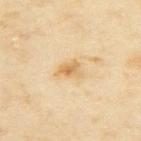Q: Was this lesion biopsied?
A: total-body-photography surveillance lesion; no biopsy
Q: What is the imaging modality?
A: ~15 mm crop, total-body skin-cancer survey
Q: Who is the patient?
A: female, roughly 35 years of age
Q: What is the anatomic site?
A: the back